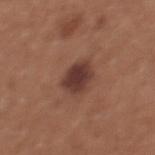The lesion was tiled from a total-body skin photograph and was not biopsied.
Cropped from a whole-body photographic skin survey; the tile spans about 15 mm.
The tile uses white-light illumination.
From the chest.
A female patient, in their mid-30s.
The total-body-photography lesion software estimated a lesion area of about 8 mm² and two-axis asymmetry of about 0.2. The software also gave a lesion color around L≈37 a*≈21 b*≈23 in CIELAB and about 12 CIELAB-L* units darker than the surrounding skin. The analysis additionally found a border-irregularity index near 2/10 and a color-variation rating of about 2.5/10. And it measured an automated nevus-likeness rating near 55 out of 100 and a lesion-detection confidence of about 100/100.